The tile uses white-light illumination; a 15 mm close-up extracted from a 3D total-body photography capture; a male subject, roughly 65 years of age; the recorded lesion diameter is about 8 mm; on the chest:
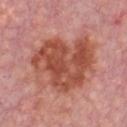  diagnosis:
    histopathology: seborrheic keratosis
    malignancy: benign
    taxonomic_path:
      - Benign
      - Benign epidermal proliferations
      - Seborrheic keratosis